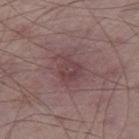The patient is a male aged 63–67. Located on the right thigh. This image is a 15 mm lesion crop taken from a total-body photograph.